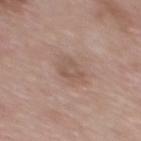Captured during whole-body skin photography for melanoma surveillance; the lesion was not biopsied. An algorithmic analysis of the crop reported an average lesion color of about L≈54 a*≈16 b*≈25 (CIELAB), roughly 7 lightness units darker than nearby skin, and a normalized lesion–skin contrast near 5. It also reported border irregularity of about 3 on a 0–10 scale, a color-variation rating of about 3/10, and peripheral color asymmetry of about 1. And it measured a classifier nevus-likeness of about 0/100 and lesion-presence confidence of about 100/100. A 15 mm crop from a total-body photograph taken for skin-cancer surveillance. Measured at roughly 3 mm in maximum diameter. On the upper back. Captured under white-light illumination. A female patient, aged approximately 50.